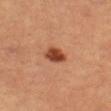The subject is a male about 60 years old. A close-up tile cropped from a whole-body skin photograph, about 15 mm across. Located on the leg. Imaged with cross-polarized lighting. The recorded lesion diameter is about 3 mm.